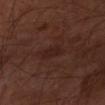Impression:
This lesion was catalogued during total-body skin photography and was not selected for biopsy.
Image and clinical context:
A 15 mm close-up tile from a total-body photography series done for melanoma screening. Captured under cross-polarized illumination. From the left forearm.